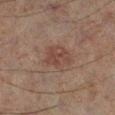This lesion was catalogued during total-body skin photography and was not selected for biopsy. Cropped from a whole-body photographic skin survey; the tile spans about 15 mm. A male patient approximately 60 years of age. Located on the left lower leg. The lesion-visualizer software estimated an average lesion color of about L≈32 a*≈15 b*≈19 (CIELAB). The analysis additionally found a border-irregularity rating of about 2/10, a color-variation rating of about 3/10, and a peripheral color-asymmetry measure near 1. And it measured a classifier nevus-likeness of about 10/100 and lesion-presence confidence of about 100/100. This is a cross-polarized tile.